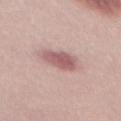This lesion was catalogued during total-body skin photography and was not selected for biopsy.
Captured under white-light illumination.
Automated tile analysis of the lesion measured an average lesion color of about L≈58 a*≈22 b*≈20 (CIELAB) and a normalized lesion–skin contrast near 8. The software also gave border irregularity of about 2 on a 0–10 scale and internal color variation of about 3 on a 0–10 scale.
A male patient about 30 years old.
A roughly 15 mm field-of-view crop from a total-body skin photograph.
The lesion's longest dimension is about 4.5 mm.